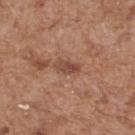The lesion was photographed on a routine skin check and not biopsied; there is no pathology result. A close-up tile cropped from a whole-body skin photograph, about 15 mm across. Captured under white-light illumination. Longest diameter approximately 3.5 mm. The total-body-photography lesion software estimated an automated nevus-likeness rating near 0 out of 100 and lesion-presence confidence of about 100/100. A male subject, aged around 65. The lesion is located on the upper back.